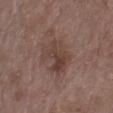Case summary:
* notes · total-body-photography surveillance lesion; no biopsy
* acquisition · total-body-photography crop, ~15 mm field of view
* anatomic site · the arm
* lesion diameter · about 5 mm
* tile lighting · white-light illumination
* subject · female, approximately 70 years of age